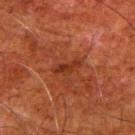| key | value |
|---|---|
| notes | catalogued during a skin exam; not biopsied |
| subject | male, about 80 years old |
| lesion size | ~3.5 mm (longest diameter) |
| tile lighting | cross-polarized |
| acquisition | total-body-photography crop, ~15 mm field of view |
| site | the right upper arm |